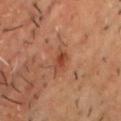Assessment: No biopsy was performed on this lesion — it was imaged during a full skin examination and was not determined to be concerning. Context: Measured at roughly 2.5 mm in maximum diameter. This is a cross-polarized tile. From the chest. A male subject, aged around 50. A 15 mm close-up extracted from a 3D total-body photography capture.